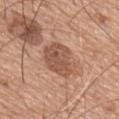* biopsy status · total-body-photography surveillance lesion; no biopsy
* image source · 15 mm crop, total-body photography
* TBP lesion metrics · about 11 CIELAB-L* units darker than the surrounding skin and a lesion-to-skin contrast of about 7.5 (normalized; higher = more distinct)
* location · the mid back
* tile lighting · white-light illumination
* size · ~5.5 mm (longest diameter)
* subject · male, in their mid-60s